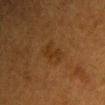biopsy_status: not biopsied; imaged during a skin examination
site: chest
lighting: cross-polarized
patient:
  sex: female
  age_approx: 40
lesion_size:
  long_diameter_mm_approx: 3.5
image:
  source: total-body photography crop
  field_of_view_mm: 15